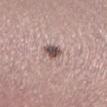No biopsy was performed on this lesion — it was imaged during a full skin examination and was not determined to be concerning.
The lesion is on the leg.
Cropped from a total-body skin-imaging series; the visible field is about 15 mm.
The subject is a male aged approximately 45.
The recorded lesion diameter is about 3 mm.